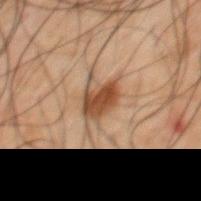automated metrics: a footprint of about 6.5 mm² and an eccentricity of roughly 0.75; a border-irregularity rating of about 2.5/10, a color-variation rating of about 3/10, and radial color variation of about 1
location: the mid back
lesion size: ~3.5 mm (longest diameter)
subject: male, about 50 years old
image: ~15 mm crop, total-body skin-cancer survey